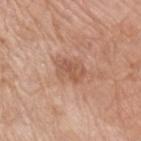Recorded during total-body skin imaging; not selected for excision or biopsy. This is a white-light tile. Longest diameter approximately 3.5 mm. Cropped from a total-body skin-imaging series; the visible field is about 15 mm. From the right upper arm. A female subject, in their mid-70s.